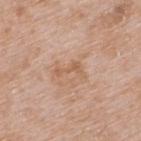Image and clinical context:
Cropped from a total-body skin-imaging series; the visible field is about 15 mm. The tile uses white-light illumination. Measured at roughly 3.5 mm in maximum diameter. The lesion is located on the back. A male subject aged 63–67.